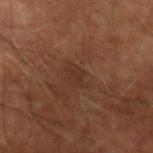notes = catalogued during a skin exam; not biopsied
site = the right forearm
imaging modality = 15 mm crop, total-body photography
tile lighting = cross-polarized
subject = male, approximately 65 years of age
size = ≈2.5 mm